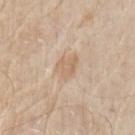workup: no biopsy performed (imaged during a skin exam)
acquisition: ~15 mm tile from a whole-body skin photo
TBP lesion metrics: a border-irregularity rating of about 3/10 and a peripheral color-asymmetry measure near 0.5; a nevus-likeness score of about 35/100 and a detector confidence of about 100 out of 100 that the crop contains a lesion
lesion size: about 3 mm
lighting: white-light
anatomic site: the arm
subject: male, aged 63–67